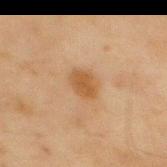{"biopsy_status": "not biopsied; imaged during a skin examination", "patient": {"sex": "male", "age_approx": 45}, "site": "mid back", "image": {"source": "total-body photography crop", "field_of_view_mm": 15}}